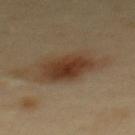The subject is a male aged 38 to 42.
Approximately 6.5 mm at its widest.
Cropped from a whole-body photographic skin survey; the tile spans about 15 mm.
Automated image analysis of the tile measured a footprint of about 20 mm², an eccentricity of roughly 0.8, and two-axis asymmetry of about 0.25. It also reported a classifier nevus-likeness of about 95/100 and lesion-presence confidence of about 100/100.
On the upper back.